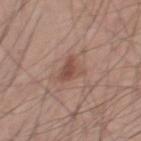workup=catalogued during a skin exam; not biopsied
location=the right thigh
image-analysis metrics=a nevus-likeness score of about 75/100 and a lesion-detection confidence of about 100/100
patient=male, approximately 45 years of age
image=total-body-photography crop, ~15 mm field of view